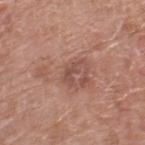{"lighting": "white-light", "lesion_size": {"long_diameter_mm_approx": 3.0}, "patient": {"sex": "male", "age_approx": 80}, "site": "leg", "image": {"source": "total-body photography crop", "field_of_view_mm": 15}, "automated_metrics": {"area_mm2_approx": 3.5, "shape_asymmetry": 0.55, "cielab_L": 49, "cielab_a": 22, "cielab_b": 25, "vs_skin_darker_L": 8.0, "border_irregularity_0_10": 7.5, "color_variation_0_10": 0.0, "peripheral_color_asymmetry": 0.0, "nevus_likeness_0_100": 0, "lesion_detection_confidence_0_100": 100}}